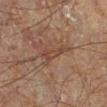Notes:
– imaging modality: ~15 mm tile from a whole-body skin photo
– tile lighting: cross-polarized illumination
– automated lesion analysis: an area of roughly 4 mm², a shape eccentricity near 0.95, and two-axis asymmetry of about 0.5; border irregularity of about 5.5 on a 0–10 scale, a within-lesion color-variation index near 1.5/10, and a peripheral color-asymmetry measure near 0.5; a classifier nevus-likeness of about 0/100 and a detector confidence of about 70 out of 100 that the crop contains a lesion
– patient: male, aged around 60
– site: the left leg
– size: ≈4 mm